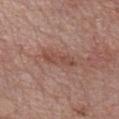Assessment: Captured during whole-body skin photography for melanoma surveillance; the lesion was not biopsied. Acquisition and patient details: About 4.5 mm across. Automated tile analysis of the lesion measured two-axis asymmetry of about 0.2. It also reported a border-irregularity index near 2.5/10 and peripheral color asymmetry of about 1. The software also gave an automated nevus-likeness rating near 0 out of 100. This is a white-light tile. A male patient roughly 70 years of age. Located on the chest. A 15 mm close-up extracted from a 3D total-body photography capture.